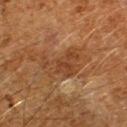{"biopsy_status": "not biopsied; imaged during a skin examination", "site": "left forearm", "image": {"source": "total-body photography crop", "field_of_view_mm": 15}, "lesion_size": {"long_diameter_mm_approx": 5.0}, "patient": {"sex": "male", "age_approx": 60}, "lighting": "cross-polarized"}